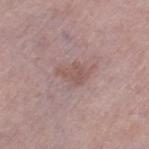Clinical impression: Recorded during total-body skin imaging; not selected for excision or biopsy. Background: The subject is a female approximately 50 years of age. Captured under white-light illumination. A lesion tile, about 15 mm wide, cut from a 3D total-body photograph. The lesion is located on the left thigh.